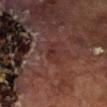notes = catalogued during a skin exam; not biopsied
tile lighting = cross-polarized
image-analysis metrics = an average lesion color of about L≈24 a*≈19 b*≈18 (CIELAB) and roughly 5 lightness units darker than nearby skin; a border-irregularity index near 3.5/10 and a within-lesion color-variation index near 2.5/10; a classifier nevus-likeness of about 0/100 and a detector confidence of about 100 out of 100 that the crop contains a lesion
patient = male, aged around 70
lesion size = ~2.5 mm (longest diameter)
acquisition = total-body-photography crop, ~15 mm field of view
location = the right lower leg